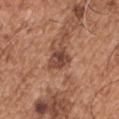The lesion was tiled from a total-body skin photograph and was not biopsied. On the front of the torso. The recorded lesion diameter is about 3.5 mm. A lesion tile, about 15 mm wide, cut from a 3D total-body photograph. The patient is a male about 55 years old. The lesion-visualizer software estimated a lesion area of about 6 mm², an eccentricity of roughly 0.7, and a symmetry-axis asymmetry near 0.3. The analysis additionally found about 12 CIELAB-L* units darker than the surrounding skin. This is a white-light tile.